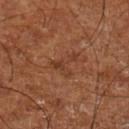biopsy_status: not biopsied; imaged during a skin examination
lighting: cross-polarized
patient:
  age_approx: 65
site: left lower leg
image:
  source: total-body photography crop
  field_of_view_mm: 15